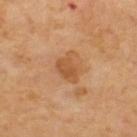Impression: Imaged during a routine full-body skin examination; the lesion was not biopsied and no histopathology is available.